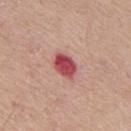Q: Is there a histopathology result?
A: no biopsy performed (imaged during a skin exam)
Q: What kind of image is this?
A: ~15 mm crop, total-body skin-cancer survey
Q: How was the tile lit?
A: white-light
Q: Automated lesion metrics?
A: a shape-asymmetry score of about 0.25 (0 = symmetric); roughly 16 lightness units darker than nearby skin; a within-lesion color-variation index near 5/10; a nevus-likeness score of about 0/100 and a detector confidence of about 100 out of 100 that the crop contains a lesion
Q: Lesion location?
A: the mid back
Q: What are the patient's age and sex?
A: male, aged around 75
Q: How large is the lesion?
A: about 3.5 mm